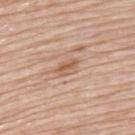Case summary:
* subject: female, aged 63 to 67
* body site: the back
* imaging modality: ~15 mm tile from a whole-body skin photo